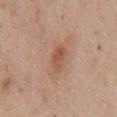Impression:
Part of a total-body skin-imaging series; this lesion was reviewed on a skin check and was not flagged for biopsy.
Context:
Automated image analysis of the tile measured a shape eccentricity near 0.8 and a shape-asymmetry score of about 0.15 (0 = symmetric). It also reported a lesion color around L≈54 a*≈21 b*≈31 in CIELAB and a lesion-to-skin contrast of about 6 (normalized; higher = more distinct). It also reported lesion-presence confidence of about 100/100. A lesion tile, about 15 mm wide, cut from a 3D total-body photograph. Imaged with white-light lighting. Measured at roughly 3 mm in maximum diameter. From the mid back. A male patient, roughly 55 years of age.